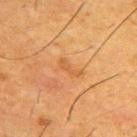imaging modality — ~15 mm tile from a whole-body skin photo; anatomic site — the upper back; subject — male, aged 33–37; lesion diameter — ~3 mm (longest diameter).